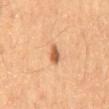No biopsy was performed on this lesion — it was imaged during a full skin examination and was not determined to be concerning.
A male subject, aged approximately 60.
Cropped from a whole-body photographic skin survey; the tile spans about 15 mm.